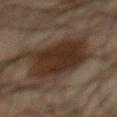  biopsy_status: not biopsied; imaged during a skin examination
  image:
    source: total-body photography crop
    field_of_view_mm: 15
  patient:
    sex: male
    age_approx: 65
  lighting: cross-polarized
  site: chest
  lesion_size:
    long_diameter_mm_approx: 8.0